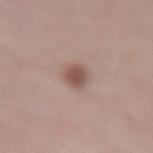| field | value |
|---|---|
| imaging modality | ~15 mm tile from a whole-body skin photo |
| tile lighting | white-light illumination |
| lesion diameter | ≈3.5 mm |
| automated lesion analysis | a lesion area of about 5.5 mm², an outline eccentricity of about 0.8 (0 = round, 1 = elongated), and a shape-asymmetry score of about 0.15 (0 = symmetric); an average lesion color of about L≈52 a*≈20 b*≈23 (CIELAB) and a lesion-to-skin contrast of about 8 (normalized; higher = more distinct); a border-irregularity index near 1.5/10 and peripheral color asymmetry of about 0.5 |
| location | the back |
| patient | female, in their mid- to late 40s |